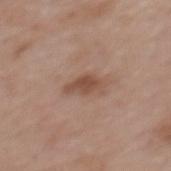Findings:
- biopsy status · imaged on a skin check; not biopsied
- imaging modality · 15 mm crop, total-body photography
- tile lighting · white-light illumination
- patient · female, aged 58–62
- location · the upper back
- size · ~4 mm (longest diameter)